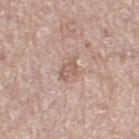{
  "lesion_size": {
    "long_diameter_mm_approx": 3.0
  },
  "site": "right thigh",
  "lighting": "white-light",
  "image": {
    "source": "total-body photography crop",
    "field_of_view_mm": 15
  },
  "automated_metrics": {
    "vs_skin_darker_L": 8.0,
    "vs_skin_contrast_norm": 5.5,
    "border_irregularity_0_10": 3.0,
    "color_variation_0_10": 2.5,
    "peripheral_color_asymmetry": 1.0
  },
  "patient": {
    "sex": "male",
    "age_approx": 65
  }
}